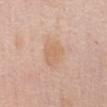{
  "biopsy_status": "not biopsied; imaged during a skin examination",
  "lighting": "white-light",
  "site": "abdomen",
  "patient": {
    "sex": "female",
    "age_approx": 60
  },
  "automated_metrics": {
    "area_mm2_approx": 9.5,
    "eccentricity": 0.6,
    "border_irregularity_0_10": 2.0,
    "color_variation_0_10": 2.0,
    "peripheral_color_asymmetry": 0.5,
    "nevus_likeness_0_100": 10,
    "lesion_detection_confidence_0_100": 100
  },
  "image": {
    "source": "total-body photography crop",
    "field_of_view_mm": 15
  },
  "lesion_size": {
    "long_diameter_mm_approx": 4.0
  }
}